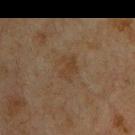biopsy status: total-body-photography surveillance lesion; no biopsy
illumination: cross-polarized illumination
location: the upper back
size: ≈3 mm
automated lesion analysis: an area of roughly 6 mm² and an eccentricity of roughly 0.55; a mean CIELAB color near L≈31 a*≈12 b*≈24 and roughly 4 lightness units darker than nearby skin
imaging modality: total-body-photography crop, ~15 mm field of view
subject: male, approximately 65 years of age